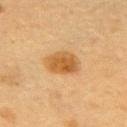Background: Measured at roughly 4 mm in maximum diameter. A 15 mm close-up tile from a total-body photography series done for melanoma screening. The subject is a female aged approximately 60. On the upper back.